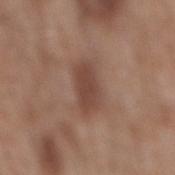{
  "biopsy_status": "not biopsied; imaged during a skin examination",
  "site": "mid back",
  "patient": {
    "sex": "male",
    "age_approx": 60
  },
  "image": {
    "source": "total-body photography crop",
    "field_of_view_mm": 15
  }
}